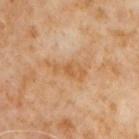notes: imaged on a skin check; not biopsied
acquisition: ~15 mm crop, total-body skin-cancer survey
automated metrics: a footprint of about 7 mm², a shape eccentricity near 0.95, and a symmetry-axis asymmetry near 0.4; about 7 CIELAB-L* units darker than the surrounding skin and a normalized border contrast of about 6; an automated nevus-likeness rating near 0 out of 100 and a lesion-detection confidence of about 100/100
lesion size: ≈5 mm
subject: male, roughly 60 years of age
site: the chest